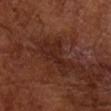The lesion was photographed on a routine skin check and not biopsied; there is no pathology result. A region of skin cropped from a whole-body photographic capture, roughly 15 mm wide. The patient is a male aged 63 to 67. The lesion is on the left arm.